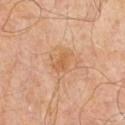The lesion was tiled from a total-body skin photograph and was not biopsied.